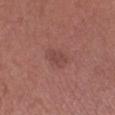<lesion>
  <lighting>white-light</lighting>
  <site>left lower leg</site>
  <patient>
    <sex>male</sex>
    <age_approx>70</age_approx>
  </patient>
  <automated_metrics>
    <cielab_L>45</cielab_L>
    <cielab_a>24</cielab_a>
    <cielab_b>23</cielab_b>
    <vs_skin_darker_L>6.0</vs_skin_darker_L>
    <vs_skin_contrast_norm>5.0</vs_skin_contrast_norm>
  </automated_metrics>
  <image>
    <source>total-body photography crop</source>
    <field_of_view_mm>15</field_of_view_mm>
  </image>
</lesion>